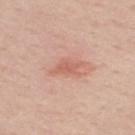follow-up=imaged on a skin check; not biopsied
image=15 mm crop, total-body photography
anatomic site=the upper back
subject=male, in their 60s
image-analysis metrics=a within-lesion color-variation index near 2/10; an automated nevus-likeness rating near 0 out of 100 and a lesion-detection confidence of about 100/100
lesion size=≈4 mm
lighting=white-light